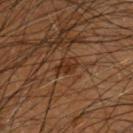Findings:
* biopsy status — no biopsy performed (imaged during a skin exam)
* location — the head or neck
* size — ≈3 mm
* subject — male, aged 83 or over
* automated lesion analysis — a lesion area of about 4 mm², an eccentricity of roughly 0.7, and two-axis asymmetry of about 0.25; an average lesion color of about L≈27 a*≈18 b*≈27 (CIELAB) and a normalized border contrast of about 7; border irregularity of about 3 on a 0–10 scale; a lesion-detection confidence of about 50/100
* acquisition — 15 mm crop, total-body photography
* lighting — cross-polarized